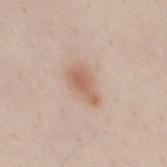{"biopsy_status": "not biopsied; imaged during a skin examination", "patient": {"sex": "male", "age_approx": 35}, "site": "back", "image": {"source": "total-body photography crop", "field_of_view_mm": 15}}